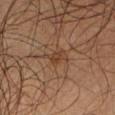Context:
The patient is a male roughly 65 years of age. Imaged with cross-polarized lighting. A 15 mm crop from a total-body photograph taken for skin-cancer surveillance. From the right forearm. Measured at roughly 3 mm in maximum diameter. The lesion-visualizer software estimated roughly 6 lightness units darker than nearby skin and a normalized lesion–skin contrast near 6. The analysis additionally found a border-irregularity rating of about 3/10, a color-variation rating of about 2/10, and radial color variation of about 1.